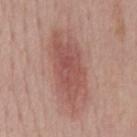The lesion was tiled from a total-body skin photograph and was not biopsied.
Cropped from a whole-body photographic skin survey; the tile spans about 15 mm.
Located on the mid back.
The patient is a male aged 53 to 57.